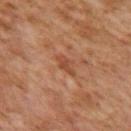Captured during whole-body skin photography for melanoma surveillance; the lesion was not biopsied. An algorithmic analysis of the crop reported a lesion area of about 3 mm², an outline eccentricity of about 0.9 (0 = round, 1 = elongated), and a symmetry-axis asymmetry near 0.4. And it measured a lesion color around L≈41 a*≈22 b*≈31 in CIELAB and a normalized border contrast of about 6.5. A female patient aged approximately 60. On the upper back. Imaged with cross-polarized lighting. The lesion's longest dimension is about 3 mm. A 15 mm close-up extracted from a 3D total-body photography capture.